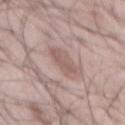Assessment:
This lesion was catalogued during total-body skin photography and was not selected for biopsy.
Image and clinical context:
A lesion tile, about 15 mm wide, cut from a 3D total-body photograph. Located on the mid back. A male patient about 45 years old.